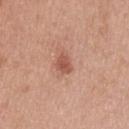Impression: Captured during whole-body skin photography for melanoma surveillance; the lesion was not biopsied. Image and clinical context: Longest diameter approximately 3 mm. The lesion is on the head or neck. A close-up tile cropped from a whole-body skin photograph, about 15 mm across. The total-body-photography lesion software estimated an area of roughly 4 mm², an outline eccentricity of about 0.75 (0 = round, 1 = elongated), and a symmetry-axis asymmetry near 0.3. The software also gave a border-irregularity index near 3/10 and a peripheral color-asymmetry measure near 0.5. Captured under white-light illumination. A male subject in their mid-40s.